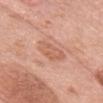workup = catalogued during a skin exam; not biopsied
body site = the head or neck
patient = female, roughly 60 years of age
lighting = white-light illumination
diameter = ≈4 mm
image source = 15 mm crop, total-body photography